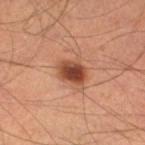Assessment: Captured during whole-body skin photography for melanoma surveillance; the lesion was not biopsied. Clinical summary: Cropped from a total-body skin-imaging series; the visible field is about 15 mm. The subject is a male in their mid-30s. On the right leg.